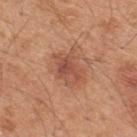notes: imaged on a skin check; not biopsied | TBP lesion metrics: roughly 9 lightness units darker than nearby skin and a normalized border contrast of about 6.5 | patient: male, aged 43–47 | acquisition: ~15 mm crop, total-body skin-cancer survey | anatomic site: the upper back | size: ≈4 mm | lighting: white-light.